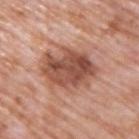The lesion was tiled from a total-body skin photograph and was not biopsied. Captured under white-light illumination. A lesion tile, about 15 mm wide, cut from a 3D total-body photograph. A male patient, about 70 years old. Approximately 6.5 mm at its widest. The lesion is located on the upper back.